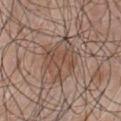Imaged during a routine full-body skin examination; the lesion was not biopsied and no histopathology is available.
A 15 mm crop from a total-body photograph taken for skin-cancer surveillance.
A male subject, aged around 50.
Located on the front of the torso.